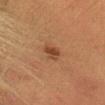Recorded during total-body skin imaging; not selected for excision or biopsy. Cropped from a whole-body photographic skin survey; the tile spans about 15 mm. The lesion is on the head or neck. The patient is a female aged 38 to 42.